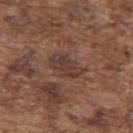Notes:
* notes: catalogued during a skin exam; not biopsied
* lesion size: about 4 mm
* subject: male, in their mid- to late 70s
* tile lighting: white-light
* image: ~15 mm tile from a whole-body skin photo
* anatomic site: the upper back